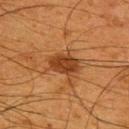The patient is a male in their mid- to late 60s. A 15 mm close-up tile from a total-body photography series done for melanoma screening. The lesion is on the upper back.